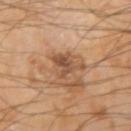{"biopsy_status": "not biopsied; imaged during a skin examination", "lesion_size": {"long_diameter_mm_approx": 3.5}, "lighting": "cross-polarized", "image": {"source": "total-body photography crop", "field_of_view_mm": 15}, "site": "right upper arm", "patient": {"sex": "male", "age_approx": 65}, "automated_metrics": {"area_mm2_approx": 7.0, "eccentricity": 0.5, "shape_asymmetry": 0.5, "cielab_L": 51, "cielab_a": 20, "cielab_b": 33, "border_irregularity_0_10": 6.0, "color_variation_0_10": 4.5, "peripheral_color_asymmetry": 1.5, "nevus_likeness_0_100": 40, "lesion_detection_confidence_0_100": 100}}